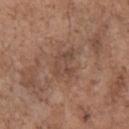notes = no biopsy performed (imaged during a skin exam); subject = male, roughly 70 years of age; acquisition = 15 mm crop, total-body photography; automated metrics = a nevus-likeness score of about 0/100; illumination = white-light; anatomic site = the chest.